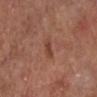diameter = ≈2.5 mm | site = the left lower leg | acquisition = total-body-photography crop, ~15 mm field of view | subject = male, aged 68–72.